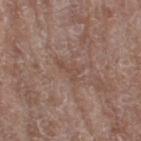Recorded during total-body skin imaging; not selected for excision or biopsy.
Measured at roughly 3.5 mm in maximum diameter.
A female subject, roughly 75 years of age.
On the right thigh.
An algorithmic analysis of the crop reported a lesion area of about 4.5 mm², a shape eccentricity near 0.85, and two-axis asymmetry of about 0.65. The software also gave border irregularity of about 8.5 on a 0–10 scale and a color-variation rating of about 0/10. And it measured an automated nevus-likeness rating near 0 out of 100 and a lesion-detection confidence of about 70/100.
Cropped from a total-body skin-imaging series; the visible field is about 15 mm.
This is a white-light tile.